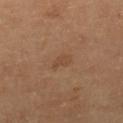The lesion was photographed on a routine skin check and not biopsied; there is no pathology result. Imaged with cross-polarized lighting. The lesion is on the right lower leg. A roughly 15 mm field-of-view crop from a total-body skin photograph. A female subject aged 58–62.